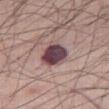Captured during whole-body skin photography for melanoma surveillance; the lesion was not biopsied.
A male patient, aged approximately 55.
From the right thigh.
A region of skin cropped from a whole-body photographic capture, roughly 15 mm wide.
Automated image analysis of the tile measured a footprint of about 9.5 mm² and a shape-asymmetry score of about 0.2 (0 = symmetric). And it measured a lesion-to-skin contrast of about 14.5 (normalized; higher = more distinct). The analysis additionally found border irregularity of about 2 on a 0–10 scale, internal color variation of about 6 on a 0–10 scale, and a peripheral color-asymmetry measure near 2. It also reported a nevus-likeness score of about 5/100 and a lesion-detection confidence of about 100/100.
The recorded lesion diameter is about 3.5 mm.